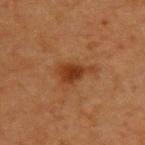Captured during whole-body skin photography for melanoma surveillance; the lesion was not biopsied. An algorithmic analysis of the crop reported a footprint of about 6 mm², an eccentricity of roughly 0.8, and two-axis asymmetry of about 0.4. The analysis additionally found a lesion color around L≈32 a*≈23 b*≈32 in CIELAB, about 9 CIELAB-L* units darker than the surrounding skin, and a lesion-to-skin contrast of about 9 (normalized; higher = more distinct). It also reported a nevus-likeness score of about 85/100 and a detector confidence of about 100 out of 100 that the crop contains a lesion. A female patient aged 38 to 42. This is a cross-polarized tile. The lesion is located on the back. A 15 mm close-up extracted from a 3D total-body photography capture. The lesion's longest dimension is about 4 mm.